A region of skin cropped from a whole-body photographic capture, roughly 15 mm wide. On the left upper arm. Longest diameter approximately 1.5 mm. A female patient aged approximately 55. Imaged with white-light lighting:
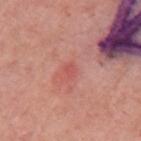On biopsy, histopathology showed a dysplastic (Clark) nevus.